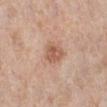{"biopsy_status": "not biopsied; imaged during a skin examination", "site": "leg", "patient": {"sex": "female", "age_approx": 65}, "lesion_size": {"long_diameter_mm_approx": 3.0}, "image": {"source": "total-body photography crop", "field_of_view_mm": 15}, "lighting": "white-light", "automated_metrics": {"area_mm2_approx": 6.0, "shape_asymmetry": 0.25, "vs_skin_darker_L": 10.0, "vs_skin_contrast_norm": 7.0}}